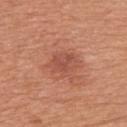  biopsy_status: not biopsied; imaged during a skin examination
  patient:
    sex: male
    age_approx: 70
  site: upper back
  lighting: white-light
  image:
    source: total-body photography crop
    field_of_view_mm: 15